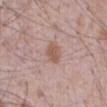biopsy status — imaged on a skin check; not biopsied
image — ~15 mm tile from a whole-body skin photo
subject — male, aged 68–72
TBP lesion metrics — a footprint of about 4.5 mm² and a shape eccentricity near 0.7; an average lesion color of about L≈56 a*≈20 b*≈26 (CIELAB), a lesion–skin lightness drop of about 9, and a lesion-to-skin contrast of about 7 (normalized; higher = more distinct); a peripheral color-asymmetry measure near 0.5; a classifier nevus-likeness of about 85/100 and lesion-presence confidence of about 100/100
lighting — white-light
anatomic site — the front of the torso
size — ~3 mm (longest diameter)